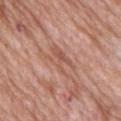Assessment: Imaged during a routine full-body skin examination; the lesion was not biopsied and no histopathology is available. Context: This image is a 15 mm lesion crop taken from a total-body photograph. Approximately 5 mm at its widest. Located on the upper back. The subject is a female about 70 years old. Captured under white-light illumination.